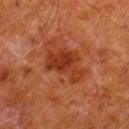Imaged with cross-polarized lighting.
Automated image analysis of the tile measured an area of roughly 12 mm², an eccentricity of roughly 0.8, and a symmetry-axis asymmetry near 0.4. The analysis additionally found an automated nevus-likeness rating near 0 out of 100 and a lesion-detection confidence of about 100/100.
The patient is a male aged 78 to 82.
A 15 mm close-up extracted from a 3D total-body photography capture.
The lesion is located on the right lower leg.